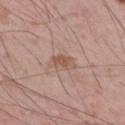Q: Is there a histopathology result?
A: total-body-photography surveillance lesion; no biopsy
Q: How was this image acquired?
A: ~15 mm crop, total-body skin-cancer survey
Q: Illumination type?
A: white-light illumination
Q: Who is the patient?
A: male, aged 53–57
Q: What is the anatomic site?
A: the right thigh
Q: How large is the lesion?
A: ~3.5 mm (longest diameter)